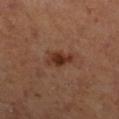biopsy status — no biopsy performed (imaged during a skin exam); image — total-body-photography crop, ~15 mm field of view; location — the right lower leg; tile lighting — cross-polarized illumination; size — ~3.5 mm (longest diameter); patient — female.